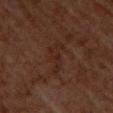The lesion was photographed on a routine skin check and not biopsied; there is no pathology result. The lesion is located on the upper back. A male patient about 60 years old. A roughly 15 mm field-of-view crop from a total-body skin photograph.